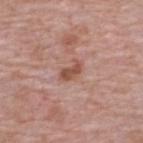Q: What is the anatomic site?
A: the upper back
Q: What are the patient's age and sex?
A: male, aged 63 to 67
Q: How was this image acquired?
A: total-body-photography crop, ~15 mm field of view
Q: How was the tile lit?
A: white-light
Q: What did automated image analysis measure?
A: an area of roughly 4.5 mm², a shape eccentricity near 0.85, and two-axis asymmetry of about 0.3; a normalized border contrast of about 7.5; a border-irregularity rating of about 3.5/10 and internal color variation of about 1.5 on a 0–10 scale; a nevus-likeness score of about 30/100 and a detector confidence of about 100 out of 100 that the crop contains a lesion
Q: What is the lesion's diameter?
A: ≈3 mm